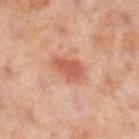Clinical impression: Captured during whole-body skin photography for melanoma surveillance; the lesion was not biopsied. Clinical summary: A region of skin cropped from a whole-body photographic capture, roughly 15 mm wide. The patient is a female in their mid- to late 50s. This is a cross-polarized tile. On the right lower leg. Measured at roughly 4 mm in maximum diameter.